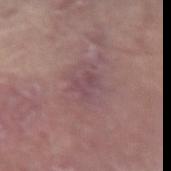Captured during whole-body skin photography for melanoma surveillance; the lesion was not biopsied.
Measured at roughly 2.5 mm in maximum diameter.
A close-up tile cropped from a whole-body skin photograph, about 15 mm across.
A male patient about 65 years old.
Located on the right forearm.